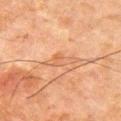Clinical impression: Imaged during a routine full-body skin examination; the lesion was not biopsied and no histopathology is available. Acquisition and patient details: Cropped from a total-body skin-imaging series; the visible field is about 15 mm. This is a cross-polarized tile. The lesion-visualizer software estimated an average lesion color of about L≈50 a*≈21 b*≈32 (CIELAB) and about 6 CIELAB-L* units darker than the surrounding skin. The software also gave a border-irregularity index near 5/10 and a within-lesion color-variation index near 2.5/10. The lesion is located on the right upper arm. A male subject, aged 63–67. About 4 mm across.